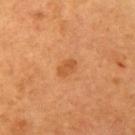{
  "biopsy_status": "not biopsied; imaged during a skin examination"
}